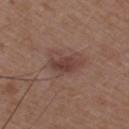The lesion was tiled from a total-body skin photograph and was not biopsied.
An algorithmic analysis of the crop reported a mean CIELAB color near L≈40 a*≈19 b*≈23, a lesion–skin lightness drop of about 9, and a normalized lesion–skin contrast near 7.5. The software also gave border irregularity of about 3 on a 0–10 scale, a color-variation rating of about 2.5/10, and a peripheral color-asymmetry measure near 1. And it measured a classifier nevus-likeness of about 35/100 and a lesion-detection confidence of about 100/100.
A close-up tile cropped from a whole-body skin photograph, about 15 mm across.
A male subject, roughly 50 years of age.
Approximately 3.5 mm at its widest.
This is a white-light tile.
Located on the right upper arm.